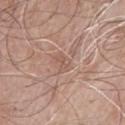The lesion was tiled from a total-body skin photograph and was not biopsied. The lesion's longest dimension is about 2.5 mm. A lesion tile, about 15 mm wide, cut from a 3D total-body photograph. Captured under white-light illumination. From the chest. A male patient aged around 65.